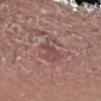- follow-up: catalogued during a skin exam; not biopsied
- imaging modality: ~15 mm tile from a whole-body skin photo
- location: the arm
- patient: male, roughly 50 years of age